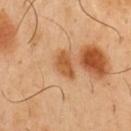notes = imaged on a skin check; not biopsied | patient = male, in their 50s | image source = 15 mm crop, total-body photography | location = the chest.